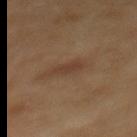| feature | finding |
|---|---|
| workup | catalogued during a skin exam; not biopsied |
| subject | male, aged 68–72 |
| imaging modality | ~15 mm tile from a whole-body skin photo |
| lesion size | ~3 mm (longest diameter) |
| automated lesion analysis | a border-irregularity index near 2.5/10, a within-lesion color-variation index near 1/10, and a peripheral color-asymmetry measure near 0.5 |
| body site | the upper back |
| illumination | cross-polarized |